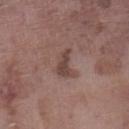<record>
  <biopsy_status>not biopsied; imaged during a skin examination</biopsy_status>
  <site>left lower leg</site>
  <patient>
    <sex>male</sex>
    <age_approx>75</age_approx>
  </patient>
  <automated_metrics>
    <cielab_L>43</cielab_L>
    <cielab_a>18</cielab_a>
    <cielab_b>21</cielab_b>
    <vs_skin_darker_L>10.0</vs_skin_darker_L>
    <vs_skin_contrast_norm>7.5</vs_skin_contrast_norm>
  </automated_metrics>
  <image>
    <source>total-body photography crop</source>
    <field_of_view_mm>15</field_of_view_mm>
  </image>
</record>